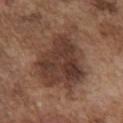  biopsy_status: not biopsied; imaged during a skin examination